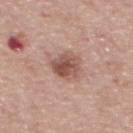Assessment: Captured during whole-body skin photography for melanoma surveillance; the lesion was not biopsied. Clinical summary: Automated tile analysis of the lesion measured a footprint of about 8.5 mm², a shape eccentricity near 0.75, and two-axis asymmetry of about 0.25. The software also gave a lesion color around L≈52 a*≈22 b*≈25 in CIELAB, roughly 13 lightness units darker than nearby skin, and a normalized lesion–skin contrast near 9. The analysis additionally found a border-irregularity index near 2.5/10, internal color variation of about 5.5 on a 0–10 scale, and peripheral color asymmetry of about 2. It also reported an automated nevus-likeness rating near 75 out of 100 and a lesion-detection confidence of about 100/100. Captured under white-light illumination. Measured at roughly 4 mm in maximum diameter. A female subject, aged 63 to 67. The lesion is located on the back. This image is a 15 mm lesion crop taken from a total-body photograph.